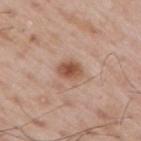Recorded during total-body skin imaging; not selected for excision or biopsy.
A region of skin cropped from a whole-body photographic capture, roughly 15 mm wide.
The lesion is on the right upper arm.
Captured under white-light illumination.
A male patient approximately 65 years of age.
Approximately 3 mm at its widest.
Automated tile analysis of the lesion measured an eccentricity of roughly 0.7 and a symmetry-axis asymmetry near 0.2. The analysis additionally found a border-irregularity rating of about 1.5/10, internal color variation of about 3.5 on a 0–10 scale, and peripheral color asymmetry of about 1.